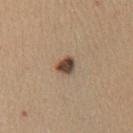– follow-up — imaged on a skin check; not biopsied
– lighting — white-light illumination
– image source — ~15 mm crop, total-body skin-cancer survey
– diameter — ~2 mm (longest diameter)
– anatomic site — the chest
– subject — female, about 60 years old